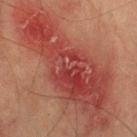biopsy status — total-body-photography surveillance lesion; no biopsy
subject — male, aged approximately 75
tile lighting — cross-polarized
image — ~15 mm crop, total-body skin-cancer survey
body site — the arm
TBP lesion metrics — a lesion color around L≈38 a*≈27 b*≈25 in CIELAB, about 9 CIELAB-L* units darker than the surrounding skin, and a normalized lesion–skin contrast near 7.5